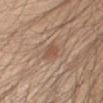The lesion was photographed on a routine skin check and not biopsied; there is no pathology result. The total-body-photography lesion software estimated an area of roughly 4.5 mm² and a symmetry-axis asymmetry near 0.25. The analysis additionally found a border-irregularity index near 2.5/10 and internal color variation of about 1.5 on a 0–10 scale. The lesion is located on the arm. Imaged with white-light lighting. A region of skin cropped from a whole-body photographic capture, roughly 15 mm wide. A male patient in their mid-30s.